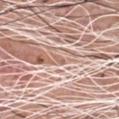{
  "biopsy_status": "not biopsied; imaged during a skin examination",
  "site": "chest",
  "image": {
    "source": "total-body photography crop",
    "field_of_view_mm": 15
  },
  "lighting": "white-light",
  "lesion_size": {
    "long_diameter_mm_approx": 2.0
  },
  "patient": {
    "sex": "male",
    "age_approx": 60
  },
  "automated_metrics": {
    "color_variation_0_10": 0.0,
    "peripheral_color_asymmetry": 0.0,
    "nevus_likeness_0_100": 0,
    "lesion_detection_confidence_0_100": 0
  }
}